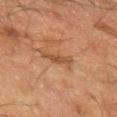Q: Was a biopsy performed?
A: catalogued during a skin exam; not biopsied
Q: Lesion size?
A: ≈3.5 mm
Q: What are the patient's age and sex?
A: male, in their 60s
Q: What is the anatomic site?
A: the left forearm
Q: What did automated image analysis measure?
A: an outline eccentricity of about 0.9 (0 = round, 1 = elongated) and a symmetry-axis asymmetry near 0.3; an average lesion color of about L≈38 a*≈17 b*≈28 (CIELAB), a lesion–skin lightness drop of about 7, and a lesion-to-skin contrast of about 6 (normalized; higher = more distinct); a border-irregularity rating of about 4/10 and peripheral color asymmetry of about 0.5
Q: What kind of image is this?
A: ~15 mm tile from a whole-body skin photo
Q: What lighting was used for the tile?
A: cross-polarized illumination